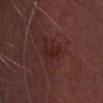The lesion was photographed on a routine skin check and not biopsied; there is no pathology result. From the head or neck. Measured at roughly 4 mm in maximum diameter. Captured under cross-polarized illumination. The subject is a male aged approximately 50. Cropped from a whole-body photographic skin survey; the tile spans about 15 mm. Automated image analysis of the tile measured a lesion color around L≈23 a*≈22 b*≈21 in CIELAB, roughly 5 lightness units darker than nearby skin, and a normalized lesion–skin contrast near 6.5. It also reported a border-irregularity index near 3.5/10 and radial color variation of about 0.5.